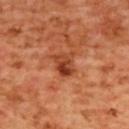Assessment:
Recorded during total-body skin imaging; not selected for excision or biopsy.
Acquisition and patient details:
The tile uses cross-polarized illumination. Longest diameter approximately 3 mm. A 15 mm crop from a total-body photograph taken for skin-cancer surveillance. The lesion is on the back. A female subject, roughly 55 years of age.